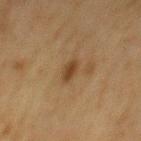subject — male, in their mid- to late 70s
TBP lesion metrics — an outline eccentricity of about 0.85 (0 = round, 1 = elongated); border irregularity of about 2 on a 0–10 scale, a color-variation rating of about 1.5/10, and radial color variation of about 0.5
image — total-body-photography crop, ~15 mm field of view
lesion diameter — ≈2.5 mm
tile lighting — cross-polarized illumination
body site — the back